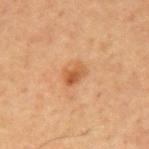Notes:
– biopsy status: total-body-photography surveillance lesion; no biopsy
– acquisition: ~15 mm tile from a whole-body skin photo
– illumination: cross-polarized illumination
– patient: male, aged 58 to 62
– size: about 2.5 mm
– site: the chest
– automated metrics: a footprint of about 4.5 mm², a shape eccentricity near 0.75, and a shape-asymmetry score of about 0.3 (0 = symmetric); a lesion color around L≈51 a*≈24 b*≈37 in CIELAB, about 9 CIELAB-L* units darker than the surrounding skin, and a normalized lesion–skin contrast near 7.5; peripheral color asymmetry of about 1.5; a classifier nevus-likeness of about 70/100 and lesion-presence confidence of about 100/100